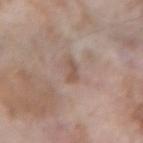notes=imaged on a skin check; not biopsied.